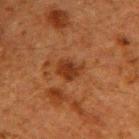The lesion was photographed on a routine skin check and not biopsied; there is no pathology result. Imaged with cross-polarized lighting. From the right upper arm. A 15 mm close-up extracted from a 3D total-body photography capture. The patient is a male aged approximately 60. The recorded lesion diameter is about 3 mm.